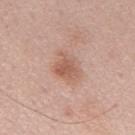The lesion was tiled from a total-body skin photograph and was not biopsied. This image is a 15 mm lesion crop taken from a total-body photograph. The lesion is on the mid back. A male subject, about 55 years old.